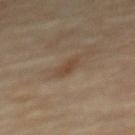Notes:
- biopsy status · imaged on a skin check; not biopsied
- imaging modality · ~15 mm crop, total-body skin-cancer survey
- lesion size · about 2.5 mm
- site · the mid back
- image-analysis metrics · a footprint of about 3 mm², a shape eccentricity near 0.85, and a shape-asymmetry score of about 0.3 (0 = symmetric); a mean CIELAB color near L≈42 a*≈14 b*≈28, about 7 CIELAB-L* units darker than the surrounding skin, and a normalized border contrast of about 6.5; a nevus-likeness score of about 0/100 and a lesion-detection confidence of about 100/100
- subject · male, roughly 85 years of age or older
- lighting · cross-polarized illumination